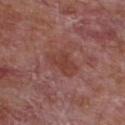Q: Is there a histopathology result?
A: catalogued during a skin exam; not biopsied
Q: What did automated image analysis measure?
A: a border-irregularity rating of about 3/10, a color-variation rating of about 2.5/10, and radial color variation of about 1
Q: What kind of image is this?
A: ~15 mm crop, total-body skin-cancer survey
Q: Lesion location?
A: the chest
Q: Patient demographics?
A: male, aged 63–67